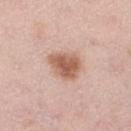Q: Lesion location?
A: the right lower leg
Q: What are the patient's age and sex?
A: female, in their mid-60s
Q: What is the imaging modality?
A: ~15 mm crop, total-body skin-cancer survey